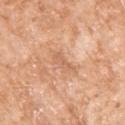Impression: Part of a total-body skin-imaging series; this lesion was reviewed on a skin check and was not flagged for biopsy. Clinical summary: A female patient in their mid- to late 70s. About 3.5 mm across. Imaged with white-light lighting. Located on the left upper arm. Cropped from a total-body skin-imaging series; the visible field is about 15 mm.